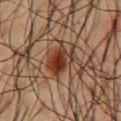biopsy status = total-body-photography surveillance lesion; no biopsy | lighting = cross-polarized illumination | lesion diameter = about 4 mm | subject = male, aged 58 to 62 | anatomic site = the chest | acquisition = ~15 mm tile from a whole-body skin photo.